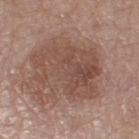workup: catalogued during a skin exam; not biopsied
patient: female, approximately 65 years of age
tile lighting: white-light
location: the right thigh
size: ≈7.5 mm
acquisition: ~15 mm tile from a whole-body skin photo